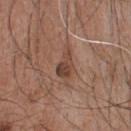The lesion was tiled from a total-body skin photograph and was not biopsied.